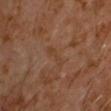Imaged during a routine full-body skin examination; the lesion was not biopsied and no histopathology is available. Cropped from a total-body skin-imaging series; the visible field is about 15 mm. The total-body-photography lesion software estimated an area of roughly 2.5 mm², a shape eccentricity near 0.95, and a shape-asymmetry score of about 0.45 (0 = symmetric). The software also gave a lesion color around L≈37 a*≈18 b*≈28 in CIELAB and about 4 CIELAB-L* units darker than the surrounding skin. The analysis additionally found a lesion-detection confidence of about 100/100. The tile uses cross-polarized illumination. A male patient, aged around 60. Located on the chest.